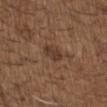Q: Was this lesion biopsied?
A: total-body-photography surveillance lesion; no biopsy
Q: How was the tile lit?
A: white-light illumination
Q: What is the imaging modality?
A: total-body-photography crop, ~15 mm field of view
Q: What are the patient's age and sex?
A: male, approximately 50 years of age
Q: Where on the body is the lesion?
A: the chest
Q: How large is the lesion?
A: ≈2.5 mm